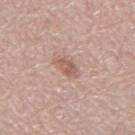Q: Was a biopsy performed?
A: catalogued during a skin exam; not biopsied
Q: Who is the patient?
A: male, in their 70s
Q: Where on the body is the lesion?
A: the mid back
Q: Illumination type?
A: white-light illumination
Q: What is the imaging modality?
A: 15 mm crop, total-body photography
Q: Automated lesion metrics?
A: a footprint of about 3.5 mm², an eccentricity of roughly 0.8, and two-axis asymmetry of about 0.25; a mean CIELAB color near L≈57 a*≈21 b*≈25, a lesion–skin lightness drop of about 10, and a lesion-to-skin contrast of about 6.5 (normalized; higher = more distinct); a border-irregularity index near 2.5/10 and peripheral color asymmetry of about 1; a nevus-likeness score of about 25/100 and a lesion-detection confidence of about 100/100